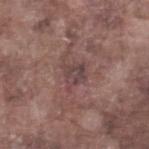The lesion was tiled from a total-body skin photograph and was not biopsied.
A male subject, aged 73 to 77.
A 15 mm crop from a total-body photograph taken for skin-cancer surveillance.
From the right lower leg.